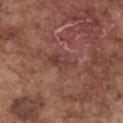Q: Was this lesion biopsied?
A: imaged on a skin check; not biopsied